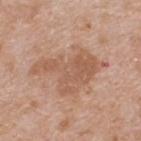image source=15 mm crop, total-body photography; location=the upper back; subject=male, about 65 years old.